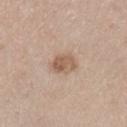  biopsy_status: not biopsied; imaged during a skin examination
  image:
    source: total-body photography crop
    field_of_view_mm: 15
  automated_metrics:
    area_mm2_approx: 6.5
    eccentricity: 0.7
    shape_asymmetry: 0.25
    border_irregularity_0_10: 2.0
    color_variation_0_10: 4.5
    peripheral_color_asymmetry: 1.5
    nevus_likeness_0_100: 25
    lesion_detection_confidence_0_100: 100
  lesion_size:
    long_diameter_mm_approx: 3.5
  patient:
    sex: female
    age_approx: 40
  site: right lower leg
  lighting: white-light